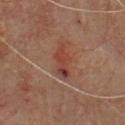patient:
  sex: male
  age_approx: 65
automated_metrics:
  area_mm2_approx: 6.0
  eccentricity: 0.9
  cielab_L: 41
  cielab_a: 25
  cielab_b: 28
  vs_skin_contrast_norm: 7.0
  border_irregularity_0_10: 4.5
  color_variation_0_10: 5.5
  lesion_detection_confidence_0_100: 95
site: front of the torso
lesion_size:
  long_diameter_mm_approx: 4.5
image:
  source: total-body photography crop
  field_of_view_mm: 15